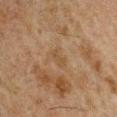Case summary:
- workup · no biopsy performed (imaged during a skin exam)